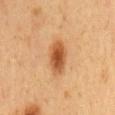No biopsy was performed on this lesion — it was imaged during a full skin examination and was not determined to be concerning.
A roughly 15 mm field-of-view crop from a total-body skin photograph.
A male subject, in their 60s.
Measured at roughly 4.5 mm in maximum diameter.
From the mid back.